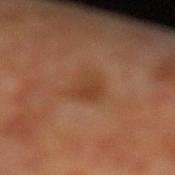A male subject aged around 60. The tile uses cross-polarized illumination. The lesion's longest dimension is about 4 mm. From the left lower leg. A close-up tile cropped from a whole-body skin photograph, about 15 mm across.